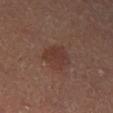Impression: Captured during whole-body skin photography for melanoma surveillance; the lesion was not biopsied. Clinical summary: A roughly 15 mm field-of-view crop from a total-body skin photograph. The lesion is on the left lower leg. Captured under cross-polarized illumination. Automated image analysis of the tile measured a lesion area of about 6.5 mm² and a shape-asymmetry score of about 0.4 (0 = symmetric). And it measured a lesion color around L≈36 a*≈20 b*≈23 in CIELAB, about 6 CIELAB-L* units darker than the surrounding skin, and a normalized lesion–skin contrast near 6. A female patient. Longest diameter approximately 3.5 mm.